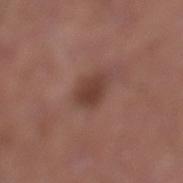Imaged during a routine full-body skin examination; the lesion was not biopsied and no histopathology is available. Imaged with white-light lighting. A male subject, aged 53 to 57. This image is a 15 mm lesion crop taken from a total-body photograph. Located on the left lower leg. The total-body-photography lesion software estimated a nevus-likeness score of about 60/100 and a detector confidence of about 100 out of 100 that the crop contains a lesion.